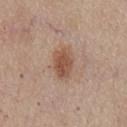Imaged during a routine full-body skin examination; the lesion was not biopsied and no histopathology is available.
Imaged with white-light lighting.
A close-up tile cropped from a whole-body skin photograph, about 15 mm across.
The lesion is on the chest.
The total-body-photography lesion software estimated an area of roughly 8 mm², an outline eccentricity of about 0.75 (0 = round, 1 = elongated), and two-axis asymmetry of about 0.2.
A male patient, aged 53–57.
About 4 mm across.